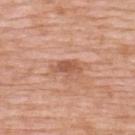The lesion was tiled from a total-body skin photograph and was not biopsied.
The lesion is on the upper back.
A roughly 15 mm field-of-view crop from a total-body skin photograph.
Automated image analysis of the tile measured an area of roughly 4.5 mm², an eccentricity of roughly 0.85, and a symmetry-axis asymmetry near 0.25. The software also gave a mean CIELAB color near L≈56 a*≈24 b*≈32, a lesion–skin lightness drop of about 10, and a normalized lesion–skin contrast near 7.
A female subject in their 70s.
The tile uses white-light illumination.
The recorded lesion diameter is about 3.5 mm.